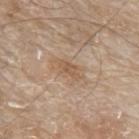Clinical impression:
Part of a total-body skin-imaging series; this lesion was reviewed on a skin check and was not flagged for biopsy.
Context:
An algorithmic analysis of the crop reported roughly 8 lightness units darker than nearby skin. It also reported a lesion-detection confidence of about 100/100. From the left forearm. The subject is a male aged approximately 80. The recorded lesion diameter is about 3.5 mm. A roughly 15 mm field-of-view crop from a total-body skin photograph. Imaged with white-light lighting.